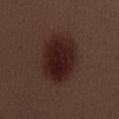The lesion was tiled from a total-body skin photograph and was not biopsied.
A female subject aged around 30.
Captured under white-light illumination.
A 15 mm crop from a total-body photograph taken for skin-cancer surveillance.
The total-body-photography lesion software estimated a lesion color around L≈21 a*≈18 b*≈18 in CIELAB and roughly 10 lightness units darker than nearby skin. The software also gave a nevus-likeness score of about 100/100 and a lesion-detection confidence of about 100/100.
Approximately 6.5 mm at its widest.
On the chest.